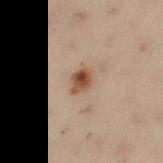Notes:
- body site · the left thigh
- lesion size · ~2.5 mm (longest diameter)
- patient · female, approximately 50 years of age
- acquisition · ~15 mm crop, total-body skin-cancer survey
- tile lighting · cross-polarized illumination